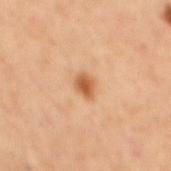Captured under cross-polarized illumination. The subject is a male aged approximately 60. The total-body-photography lesion software estimated a lesion area of about 4 mm², a shape eccentricity near 0.7, and a shape-asymmetry score of about 0.2 (0 = symmetric). The analysis additionally found a border-irregularity rating of about 2/10 and radial color variation of about 1. A close-up tile cropped from a whole-body skin photograph, about 15 mm across. The lesion is located on the back. Longest diameter approximately 2.5 mm.